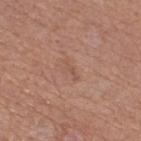follow-up: imaged on a skin check; not biopsied | patient: female, roughly 60 years of age | image source: total-body-photography crop, ~15 mm field of view | illumination: white-light | lesion diameter: about 2.5 mm | body site: the right thigh.